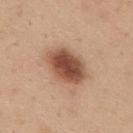<lesion>
<biopsy_status>not biopsied; imaged during a skin examination</biopsy_status>
<patient>
  <sex>male</sex>
  <age_approx>35</age_approx>
</patient>
<site>mid back</site>
<lighting>white-light</lighting>
<lesion_size>
  <long_diameter_mm_approx>5.5</long_diameter_mm_approx>
</lesion_size>
<image>
  <source>total-body photography crop</source>
  <field_of_view_mm>15</field_of_view_mm>
</image>
</lesion>